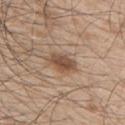| field | value |
|---|---|
| follow-up | total-body-photography surveillance lesion; no biopsy |
| location | the right upper arm |
| acquisition | ~15 mm tile from a whole-body skin photo |
| subject | male, roughly 50 years of age |
| automated lesion analysis | a lesion area of about 6 mm² and a shape eccentricity near 0.8; a color-variation rating of about 4/10 and peripheral color asymmetry of about 1.5; a nevus-likeness score of about 85/100 and a detector confidence of about 100 out of 100 that the crop contains a lesion |